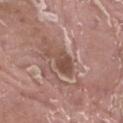Assessment:
This lesion was catalogued during total-body skin photography and was not selected for biopsy.
Clinical summary:
The subject is a male in their 40s. This image is a 15 mm lesion crop taken from a total-body photograph. On the leg.